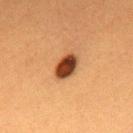  biopsy_status: not biopsied; imaged during a skin examination
  site: upper back
  image:
    source: total-body photography crop
    field_of_view_mm: 15
  patient:
    sex: female
    age_approx: 55